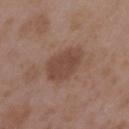Clinical impression: No biopsy was performed on this lesion — it was imaged during a full skin examination and was not determined to be concerning. Clinical summary: Captured under white-light illumination. Automated tile analysis of the lesion measured a lesion–skin lightness drop of about 9 and a normalized border contrast of about 7. The software also gave a border-irregularity rating of about 1.5/10, a within-lesion color-variation index near 2/10, and a peripheral color-asymmetry measure near 0.5. The lesion's longest dimension is about 5 mm. A female patient, roughly 35 years of age. Located on the left upper arm. A 15 mm crop from a total-body photograph taken for skin-cancer surveillance.